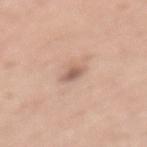follow-up: catalogued during a skin exam; not biopsied
anatomic site: the back
image: 15 mm crop, total-body photography
lesion size: ≈2.5 mm
automated metrics: a footprint of about 3 mm² and an outline eccentricity of about 0.85 (0 = round, 1 = elongated); a classifier nevus-likeness of about 65/100
patient: female, approximately 50 years of age